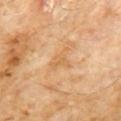| feature | finding |
|---|---|
| biopsy status | no biopsy performed (imaged during a skin exam) |
| lesion size | ≈3.5 mm |
| illumination | cross-polarized |
| image source | ~15 mm tile from a whole-body skin photo |
| automated lesion analysis | an average lesion color of about L≈61 a*≈19 b*≈40 (CIELAB), roughly 6 lightness units darker than nearby skin, and a normalized border contrast of about 5; a border-irregularity index near 5/10, a color-variation rating of about 1.5/10, and peripheral color asymmetry of about 0.5; a detector confidence of about 100 out of 100 that the crop contains a lesion |
| body site | the chest |
| subject | male, aged 58 to 62 |